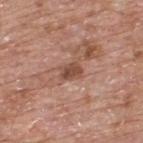Q: Was this lesion biopsied?
A: imaged on a skin check; not biopsied
Q: What is the lesion's diameter?
A: about 3 mm
Q: Where on the body is the lesion?
A: the upper back
Q: What kind of image is this?
A: total-body-photography crop, ~15 mm field of view
Q: What are the patient's age and sex?
A: male, in their mid- to late 60s
Q: What did automated image analysis measure?
A: a border-irregularity rating of about 2/10, a within-lesion color-variation index near 2.5/10, and radial color variation of about 1; a lesion-detection confidence of about 100/100